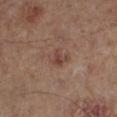No biopsy was performed on this lesion — it was imaged during a full skin examination and was not determined to be concerning. A 15 mm close-up tile from a total-body photography series done for melanoma screening. The subject is a male about 65 years old. The total-body-photography lesion software estimated a border-irregularity rating of about 3/10. The software also gave lesion-presence confidence of about 100/100. This is a cross-polarized tile. The lesion is located on the left lower leg.